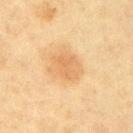The lesion was tiled from a total-body skin photograph and was not biopsied.
On the right upper arm.
This is a cross-polarized tile.
A female subject aged 53–57.
A region of skin cropped from a whole-body photographic capture, roughly 15 mm wide.